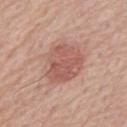{"biopsy_status": "not biopsied; imaged during a skin examination", "lesion_size": {"long_diameter_mm_approx": 5.0}, "site": "front of the torso", "patient": {"sex": "male", "age_approx": 75}, "image": {"source": "total-body photography crop", "field_of_view_mm": 15}, "automated_metrics": {"area_mm2_approx": 17.0, "eccentricity": 0.5, "shape_asymmetry": 0.2}}